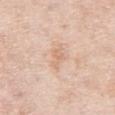{"biopsy_status": "not biopsied; imaged during a skin examination", "patient": {"sex": "male", "age_approx": 75}, "image": {"source": "total-body photography crop", "field_of_view_mm": 15}, "lighting": "white-light", "automated_metrics": {"area_mm2_approx": 3.0, "eccentricity": 0.9, "shape_asymmetry": 0.35, "cielab_L": 69, "cielab_a": 19, "cielab_b": 32, "vs_skin_darker_L": 7.0}, "site": "chest"}